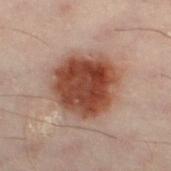A roughly 15 mm field-of-view crop from a total-body skin photograph. The total-body-photography lesion software estimated a lesion area of about 28 mm² and two-axis asymmetry of about 0.15. The analysis additionally found a border-irregularity index near 2/10 and radial color variation of about 1.5. It also reported a classifier nevus-likeness of about 100/100 and a lesion-detection confidence of about 100/100. This is a cross-polarized tile. The subject is a male about 50 years old. The lesion is on the left lower leg. Approximately 6.5 mm at its widest.